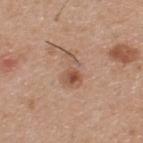Q: Was this lesion biopsied?
A: catalogued during a skin exam; not biopsied
Q: What kind of image is this?
A: total-body-photography crop, ~15 mm field of view
Q: How large is the lesion?
A: about 4 mm
Q: Lesion location?
A: the upper back
Q: What are the patient's age and sex?
A: male, approximately 60 years of age
Q: What did automated image analysis measure?
A: a border-irregularity rating of about 4.5/10, a color-variation rating of about 8.5/10, and radial color variation of about 3; an automated nevus-likeness rating near 30 out of 100 and a lesion-detection confidence of about 100/100
Q: What lighting was used for the tile?
A: white-light illumination